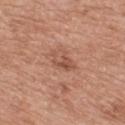The lesion was photographed on a routine skin check and not biopsied; there is no pathology result.